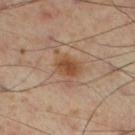A male patient, roughly 55 years of age.
Captured under cross-polarized illumination.
Cropped from a total-body skin-imaging series; the visible field is about 15 mm.
On the right lower leg.
Longest diameter approximately 5 mm.